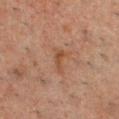Part of a total-body skin-imaging series; this lesion was reviewed on a skin check and was not flagged for biopsy. Imaged with cross-polarized lighting. Cropped from a whole-body photographic skin survey; the tile spans about 15 mm. The lesion is on the chest. The subject is a male approximately 50 years of age. Measured at roughly 3 mm in maximum diameter.